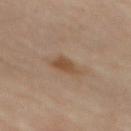| key | value |
|---|---|
| notes | catalogued during a skin exam; not biopsied |
| image-analysis metrics | a lesion area of about 4.5 mm² and an outline eccentricity of about 0.75 (0 = round, 1 = elongated); border irregularity of about 3 on a 0–10 scale, internal color variation of about 2.5 on a 0–10 scale, and peripheral color asymmetry of about 1 |
| patient | female, in their mid- to late 70s |
| acquisition | ~15 mm crop, total-body skin-cancer survey |
| anatomic site | the chest |
| lesion size | ≈3 mm |